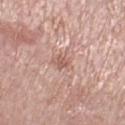Assessment: Imaged during a routine full-body skin examination; the lesion was not biopsied and no histopathology is available. Acquisition and patient details: A female patient approximately 70 years of age. From the right lower leg. A 15 mm close-up tile from a total-body photography series done for melanoma screening. The total-body-photography lesion software estimated a classifier nevus-likeness of about 0/100 and lesion-presence confidence of about 100/100. Measured at roughly 2.5 mm in maximum diameter.